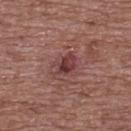notes: catalogued during a skin exam; not biopsied | patient: female, roughly 75 years of age | location: the upper back | imaging modality: 15 mm crop, total-body photography.